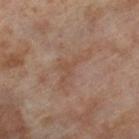Imaged during a routine full-body skin examination; the lesion was not biopsied and no histopathology is available. A close-up tile cropped from a whole-body skin photograph, about 15 mm across. Longest diameter approximately 5.5 mm. The subject is a female approximately 55 years of age. Automated image analysis of the tile measured a classifier nevus-likeness of about 0/100 and lesion-presence confidence of about 95/100. Captured under cross-polarized illumination. Located on the right thigh.